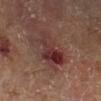{
  "biopsy_status": "not biopsied; imaged during a skin examination",
  "patient": {
    "sex": "male",
    "age_approx": 70
  },
  "automated_metrics": {
    "border_irregularity_0_10": 4.5,
    "color_variation_0_10": 9.5,
    "peripheral_color_asymmetry": 3.0,
    "nevus_likeness_0_100": 0
  },
  "lighting": "cross-polarized",
  "lesion_size": {
    "long_diameter_mm_approx": 5.5
  },
  "site": "right lower leg",
  "image": {
    "source": "total-body photography crop",
    "field_of_view_mm": 15
  }
}